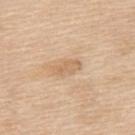{"biopsy_status": "not biopsied; imaged during a skin examination", "patient": {"sex": "female", "age_approx": 55}, "lesion_size": {"long_diameter_mm_approx": 3.0}, "image": {"source": "total-body photography crop", "field_of_view_mm": 15}, "lighting": "white-light", "site": "upper back", "automated_metrics": {"cielab_L": 65, "cielab_a": 17, "cielab_b": 36, "vs_skin_contrast_norm": 5.5, "border_irregularity_0_10": 3.5, "color_variation_0_10": 1.0, "peripheral_color_asymmetry": 0.0, "nevus_likeness_0_100": 0}}